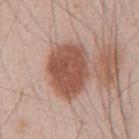{
  "biopsy_status": "not biopsied; imaged during a skin examination",
  "image": {
    "source": "total-body photography crop",
    "field_of_view_mm": 15
  },
  "patient": {
    "sex": "male",
    "age_approx": 45
  },
  "lesion_size": {
    "long_diameter_mm_approx": 6.5
  },
  "lighting": "white-light",
  "site": "abdomen"
}